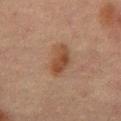Part of a total-body skin-imaging series; this lesion was reviewed on a skin check and was not flagged for biopsy. A male patient, in their mid- to late 60s. A close-up tile cropped from a whole-body skin photograph, about 15 mm across. Longest diameter approximately 5 mm. Captured under cross-polarized illumination. The lesion is located on the back.